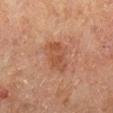– biopsy status · imaged on a skin check; not biopsied
– image · total-body-photography crop, ~15 mm field of view
– patient · male, aged around 65
– body site · the right lower leg